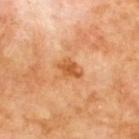workup = total-body-photography surveillance lesion; no biopsy